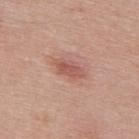Clinical impression:
No biopsy was performed on this lesion — it was imaged during a full skin examination and was not determined to be concerning.
Acquisition and patient details:
The lesion is on the upper back. A 15 mm close-up extracted from a 3D total-body photography capture. The patient is a female aged around 50.